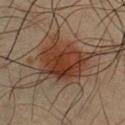Impression:
Captured during whole-body skin photography for melanoma surveillance; the lesion was not biopsied.
Acquisition and patient details:
From the chest. Measured at roughly 5.5 mm in maximum diameter. The subject is a male aged 58 to 62. Cropped from a whole-body photographic skin survey; the tile spans about 15 mm.